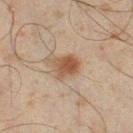{"biopsy_status": "not biopsied; imaged during a skin examination", "patient": {"sex": "male", "age_approx": 45}, "lighting": "cross-polarized", "image": {"source": "total-body photography crop", "field_of_view_mm": 15}, "site": "right thigh", "automated_metrics": {"area_mm2_approx": 6.5, "eccentricity": 0.35, "shape_asymmetry": 0.25, "color_variation_0_10": 3.5}, "lesion_size": {"long_diameter_mm_approx": 3.0}}